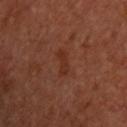image source: total-body-photography crop, ~15 mm field of view | TBP lesion metrics: a lesion area of about 3 mm², an outline eccentricity of about 0.9 (0 = round, 1 = elongated), and a symmetry-axis asymmetry near 0.4; an average lesion color of about L≈27 a*≈22 b*≈27 (CIELAB), about 5 CIELAB-L* units darker than the surrounding skin, and a lesion-to-skin contrast of about 6.5 (normalized; higher = more distinct); a border-irregularity index near 4.5/10, a within-lesion color-variation index near 0/10, and peripheral color asymmetry of about 0; a nevus-likeness score of about 0/100 and lesion-presence confidence of about 100/100 | lighting: cross-polarized | anatomic site: the chest | subject: male, aged 63 to 67.